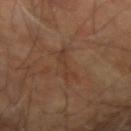  biopsy_status: not biopsied; imaged during a skin examination
  lesion_size:
    long_diameter_mm_approx: 4.0
  site: right upper arm
  image:
    source: total-body photography crop
    field_of_view_mm: 15
  automated_metrics:
    shape_asymmetry: 0.5
    cielab_L: 36
    cielab_a: 17
    cielab_b: 28
    vs_skin_contrast_norm: 5.0
    color_variation_0_10: 0.0
    peripheral_color_asymmetry: 0.0
  lighting: cross-polarized
  patient:
    sex: male
    age_approx: 70